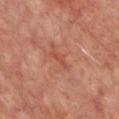<tbp_lesion>
<biopsy_status>not biopsied; imaged during a skin examination</biopsy_status>
<image>
  <source>total-body photography crop</source>
  <field_of_view_mm>15</field_of_view_mm>
</image>
<lighting>cross-polarized</lighting>
<site>front of the torso</site>
<patient>
  <age_approx>55</age_approx>
</patient>
<lesion_size>
  <long_diameter_mm_approx>3.0</long_diameter_mm_approx>
</lesion_size>
</tbp_lesion>